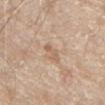Impression: Recorded during total-body skin imaging; not selected for excision or biopsy.